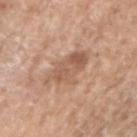The lesion was tiled from a total-body skin photograph and was not biopsied. Automated image analysis of the tile measured a lesion color around L≈57 a*≈20 b*≈30 in CIELAB, roughly 9 lightness units darker than nearby skin, and a lesion-to-skin contrast of about 6 (normalized; higher = more distinct). The software also gave a border-irregularity index near 4.5/10, internal color variation of about 4 on a 0–10 scale, and radial color variation of about 1.5. Measured at roughly 4.5 mm in maximum diameter. This image is a 15 mm lesion crop taken from a total-body photograph. Captured under white-light illumination. A male subject, about 60 years old. On the right upper arm.